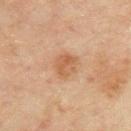follow-up: imaged on a skin check; not biopsied
subject: male, in their mid-40s
imaging modality: total-body-photography crop, ~15 mm field of view
automated lesion analysis: an average lesion color of about L≈50 a*≈19 b*≈32 (CIELAB) and about 8 CIELAB-L* units darker than the surrounding skin; a border-irregularity rating of about 2/10, a color-variation rating of about 3.5/10, and peripheral color asymmetry of about 1.5; an automated nevus-likeness rating near 5 out of 100
anatomic site: the upper back